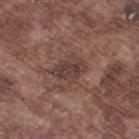Impression: Captured during whole-body skin photography for melanoma surveillance; the lesion was not biopsied. Context: A male patient, roughly 75 years of age. A 15 mm close-up extracted from a 3D total-body photography capture. Approximately 4 mm at its widest. The lesion is on the left thigh.